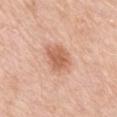subject=female, roughly 70 years of age | lesion diameter=~4 mm (longest diameter) | tile lighting=white-light | automated lesion analysis=a detector confidence of about 100 out of 100 that the crop contains a lesion | anatomic site=the mid back | imaging modality=~15 mm crop, total-body skin-cancer survey.